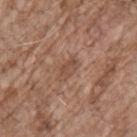  biopsy_status: not biopsied; imaged during a skin examination
  patient:
    sex: male
    age_approx: 70
  automated_metrics:
    area_mm2_approx: 4.5
    cielab_L: 49
    cielab_a: 19
    cielab_b: 28
    vs_skin_contrast_norm: 5.5
  image:
    source: total-body photography crop
    field_of_view_mm: 15
  site: chest